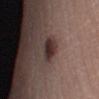Assessment: This lesion was catalogued during total-body skin photography and was not selected for biopsy. Context: The lesion-visualizer software estimated a shape eccentricity near 0.7 and a symmetry-axis asymmetry near 0.25. It also reported an automated nevus-likeness rating near 95 out of 100 and a lesion-detection confidence of about 100/100. A 15 mm crop from a total-body photograph taken for skin-cancer surveillance. The patient is a female aged around 35. Measured at roughly 3.5 mm in maximum diameter. On the left lower leg. The tile uses white-light illumination.